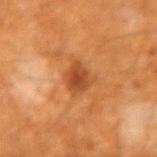Captured during whole-body skin photography for melanoma surveillance; the lesion was not biopsied. About 2.5 mm across. The lesion is on the arm. A region of skin cropped from a whole-body photographic capture, roughly 15 mm wide. Imaged with cross-polarized lighting. The patient is a male roughly 60 years of age.